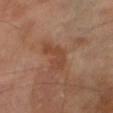Imaged during a routine full-body skin examination; the lesion was not biopsied and no histopathology is available. The patient is a male aged 68–72. This image is a 15 mm lesion crop taken from a total-body photograph. Located on the right thigh. Imaged with cross-polarized lighting.